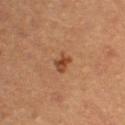Q: Was a biopsy performed?
A: no biopsy performed (imaged during a skin exam)
Q: How was the tile lit?
A: cross-polarized illumination
Q: What is the lesion's diameter?
A: ≈2.5 mm
Q: Where on the body is the lesion?
A: the right forearm
Q: What are the patient's age and sex?
A: female, aged 68–72
Q: What did automated image analysis measure?
A: an area of roughly 3.5 mm², an outline eccentricity of about 0.55 (0 = round, 1 = elongated), and a shape-asymmetry score of about 0.25 (0 = symmetric); an average lesion color of about L≈38 a*≈21 b*≈30 (CIELAB) and about 9 CIELAB-L* units darker than the surrounding skin; a border-irregularity rating of about 2.5/10, a color-variation rating of about 2.5/10, and radial color variation of about 1; an automated nevus-likeness rating near 85 out of 100 and a lesion-detection confidence of about 100/100
Q: What kind of image is this?
A: ~15 mm crop, total-body skin-cancer survey